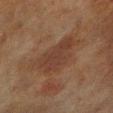workup: catalogued during a skin exam; not biopsied | subject: male, aged 68–72 | lesion diameter: ~5 mm (longest diameter) | body site: the right lower leg | image: ~15 mm crop, total-body skin-cancer survey | automated lesion analysis: an area of roughly 14 mm², a shape eccentricity near 0.75, and two-axis asymmetry of about 0.35; an average lesion color of about L≈31 a*≈17 b*≈23 (CIELAB) and a lesion–skin lightness drop of about 6; a border-irregularity index near 4/10 and peripheral color asymmetry of about 0.5; an automated nevus-likeness rating near 0 out of 100 and a lesion-detection confidence of about 100/100.